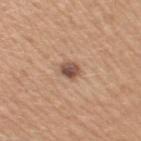subject: female, aged 28 to 32 | illumination: white-light | body site: the right upper arm | lesion diameter: ~2.5 mm (longest diameter) | imaging modality: total-body-photography crop, ~15 mm field of view.